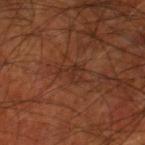The lesion was photographed on a routine skin check and not biopsied; there is no pathology result.
From the right thigh.
A male patient roughly 70 years of age.
A region of skin cropped from a whole-body photographic capture, roughly 15 mm wide.